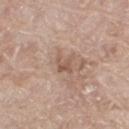* biopsy status · catalogued during a skin exam; not biopsied
* lighting · white-light illumination
* lesion diameter · about 2.5 mm
* body site · the right thigh
* patient · female, aged approximately 75
* image · ~15 mm tile from a whole-body skin photo